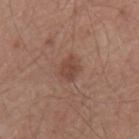| key | value |
|---|---|
| subject | male, approximately 65 years of age |
| image-analysis metrics | a lesion color around L≈45 a*≈20 b*≈26 in CIELAB and a normalized border contrast of about 6.5; lesion-presence confidence of about 100/100 |
| size | ≈2.5 mm |
| lighting | white-light |
| site | the mid back |
| image source | ~15 mm tile from a whole-body skin photo |